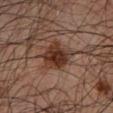Clinical impression:
The lesion was tiled from a total-body skin photograph and was not biopsied.
Image and clinical context:
Cropped from a whole-body photographic skin survey; the tile spans about 15 mm. A male subject, in their mid- to late 40s. An algorithmic analysis of the crop reported a classifier nevus-likeness of about 90/100. This is a cross-polarized tile. From the left forearm. Longest diameter approximately 3.5 mm.